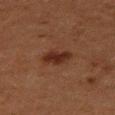Clinical impression:
The lesion was photographed on a routine skin check and not biopsied; there is no pathology result.
Context:
The total-body-photography lesion software estimated a border-irregularity index near 2.5/10. And it measured a classifier nevus-likeness of about 85/100 and a detector confidence of about 100 out of 100 that the crop contains a lesion. The lesion is on the right thigh. Cropped from a whole-body photographic skin survey; the tile spans about 15 mm. Imaged with cross-polarized lighting. Measured at roughly 3.5 mm in maximum diameter. The patient is a female aged 48 to 52.